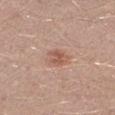{"biopsy_status": "not biopsied; imaged during a skin examination", "image": {"source": "total-body photography crop", "field_of_view_mm": 15}, "patient": {"sex": "male", "age_approx": 30}, "site": "left lower leg", "lighting": "white-light", "lesion_size": {"long_diameter_mm_approx": 2.5}}